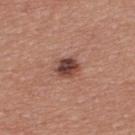The lesion is on the back. The lesion's longest dimension is about 3 mm. A male patient, aged 38 to 42. A 15 mm close-up extracted from a 3D total-body photography capture.